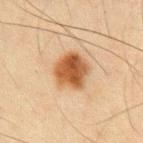– notes: no biopsy performed (imaged during a skin exam)